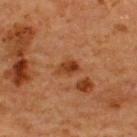Assessment:
Recorded during total-body skin imaging; not selected for excision or biopsy.
Image and clinical context:
A 15 mm crop from a total-body photograph taken for skin-cancer surveillance. From the upper back. The subject is a male in their mid-60s.